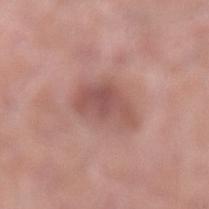{
  "biopsy_status": "not biopsied; imaged during a skin examination",
  "lighting": "white-light",
  "site": "left lower leg",
  "lesion_size": {
    "long_diameter_mm_approx": 4.5
  },
  "image": {
    "source": "total-body photography crop",
    "field_of_view_mm": 15
  },
  "patient": {
    "sex": "male",
    "age_approx": 20
  }
}